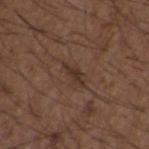follow-up = total-body-photography surveillance lesion; no biopsy | acquisition = 15 mm crop, total-body photography | subject = male, aged around 50 | location = the back.